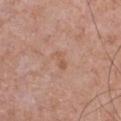The lesion was tiled from a total-body skin photograph and was not biopsied. Located on the chest. The lesion-visualizer software estimated a border-irregularity index near 3.5/10, a within-lesion color-variation index near 2/10, and peripheral color asymmetry of about 0.5. A male subject, in their mid-60s. The lesion's longest dimension is about 2.5 mm. A lesion tile, about 15 mm wide, cut from a 3D total-body photograph.